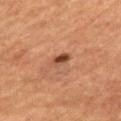  lighting: cross-polarized
  image:
    source: total-body photography crop
    field_of_view_mm: 15
  patient:
    sex: female
    age_approx: 70
  site: mid back